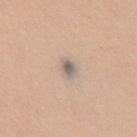biopsy status = total-body-photography surveillance lesion; no biopsy
TBP lesion metrics = a lesion area of about 3.5 mm², a shape eccentricity near 0.75, and a symmetry-axis asymmetry near 0.15; an average lesion color of about L≈61 a*≈9 b*≈21 (CIELAB) and a normalized lesion–skin contrast near 8; a border-irregularity rating of about 1.5/10, a color-variation rating of about 6/10, and peripheral color asymmetry of about 2; a classifier nevus-likeness of about 15/100 and lesion-presence confidence of about 80/100
acquisition = ~15 mm tile from a whole-body skin photo
site = the chest
patient = female, aged around 45
lighting = white-light illumination
lesion size = about 2.5 mm